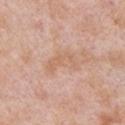No biopsy was performed on this lesion — it was imaged during a full skin examination and was not determined to be concerning. A male subject aged approximately 55. Measured at roughly 4 mm in maximum diameter. A close-up tile cropped from a whole-body skin photograph, about 15 mm across. An algorithmic analysis of the crop reported a mean CIELAB color near L≈63 a*≈20 b*≈32, roughly 6 lightness units darker than nearby skin, and a normalized border contrast of about 5. Captured under white-light illumination. On the left upper arm.